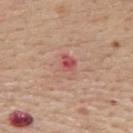This lesion was catalogued during total-body skin photography and was not selected for biopsy. The subject is a female about 65 years old. Measured at roughly 3 mm in maximum diameter. A 15 mm crop from a total-body photograph taken for skin-cancer surveillance. On the back. Imaged with white-light lighting.